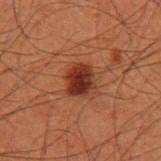Captured during whole-body skin photography for melanoma surveillance; the lesion was not biopsied.
A 15 mm close-up tile from a total-body photography series done for melanoma screening.
The tile uses cross-polarized illumination.
A male patient in their 60s.
An algorithmic analysis of the crop reported an area of roughly 8 mm² and a symmetry-axis asymmetry near 0.15.
From the left upper arm.
Approximately 3.5 mm at its widest.